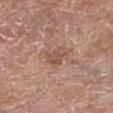Findings:
– biopsy status: catalogued during a skin exam; not biopsied
– subject: male, aged around 65
– body site: the right thigh
– image-analysis metrics: border irregularity of about 4 on a 0–10 scale and radial color variation of about 0.5; lesion-presence confidence of about 100/100
– imaging modality: ~15 mm tile from a whole-body skin photo
– size: about 2.5 mm